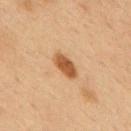Part of a total-body skin-imaging series; this lesion was reviewed on a skin check and was not flagged for biopsy. From the upper back. Captured under cross-polarized illumination. A lesion tile, about 15 mm wide, cut from a 3D total-body photograph. The subject is a male aged around 70. The lesion's longest dimension is about 3.5 mm.